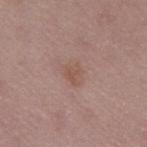Findings:
- notes · catalogued during a skin exam; not biopsied
- subject · female, approximately 50 years of age
- acquisition · 15 mm crop, total-body photography
- lesion diameter · ≈3 mm
- illumination · white-light
- site · the left thigh